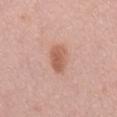Case summary:
– follow-up: imaged on a skin check; not biopsied
– lesion diameter: ~3.5 mm (longest diameter)
– lighting: white-light illumination
– site: the mid back
– image: ~15 mm crop, total-body skin-cancer survey
– subject: male, aged around 55